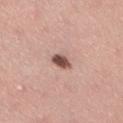Captured during whole-body skin photography for melanoma surveillance; the lesion was not biopsied. A 15 mm close-up extracted from a 3D total-body photography capture. The total-body-photography lesion software estimated a lesion area of about 3.5 mm², a shape eccentricity near 0.75, and a symmetry-axis asymmetry near 0.2. The software also gave a border-irregularity index near 1.5/10, internal color variation of about 4 on a 0–10 scale, and radial color variation of about 1.5. A female subject, aged 33 to 37. On the right thigh. The recorded lesion diameter is about 2.5 mm. The tile uses white-light illumination.